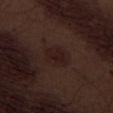This lesion was catalogued during total-body skin photography and was not selected for biopsy.
A male subject aged 68 to 72.
Cropped from a total-body skin-imaging series; the visible field is about 15 mm.
The lesion is located on the left thigh.
The tile uses white-light illumination.